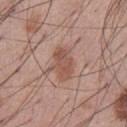Part of a total-body skin-imaging series; this lesion was reviewed on a skin check and was not flagged for biopsy. Located on the abdomen. Longest diameter approximately 4 mm. Captured under white-light illumination. A 15 mm close-up tile from a total-body photography series done for melanoma screening. A male subject aged around 55.